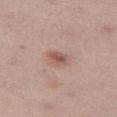notes — total-body-photography surveillance lesion; no biopsy | subject — female, in their mid-20s | image — 15 mm crop, total-body photography | lesion diameter — ≈2.5 mm | anatomic site — the left thigh | lighting — white-light illumination.